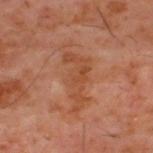Impression:
The lesion was photographed on a routine skin check and not biopsied; there is no pathology result.
Acquisition and patient details:
The patient is a male aged 58–62. Located on the upper back. A region of skin cropped from a whole-body photographic capture, roughly 15 mm wide.